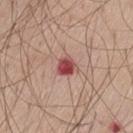tile lighting: white-light illumination
anatomic site: the left thigh
image source: total-body-photography crop, ~15 mm field of view
subject: male, aged 63–67
image-analysis metrics: a lesion area of about 5 mm², an outline eccentricity of about 0.4 (0 = round, 1 = elongated), and a shape-asymmetry score of about 0.3 (0 = symmetric); a border-irregularity index near 2.5/10 and a peripheral color-asymmetry measure near 1.5; a classifier nevus-likeness of about 0/100 and lesion-presence confidence of about 100/100
lesion size: ≈2.5 mm